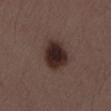site: lower back
patient:
  sex: male
  age_approx: 30
image:
  source: total-body photography crop
  field_of_view_mm: 15